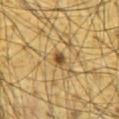Findings:
- workup — imaged on a skin check; not biopsied
- imaging modality — total-body-photography crop, ~15 mm field of view
- site — the abdomen
- subject — male, in their mid- to late 60s